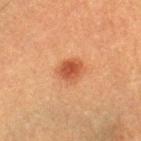Findings:
- follow-up: total-body-photography surveillance lesion; no biopsy
- patient: male, approximately 40 years of age
- image source: total-body-photography crop, ~15 mm field of view
- body site: the right lower leg